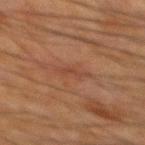notes: no biopsy performed (imaged during a skin exam) | anatomic site: the back | image source: 15 mm crop, total-body photography | size: ~2.5 mm (longest diameter) | patient: male, aged around 65 | tile lighting: cross-polarized.